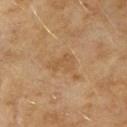Clinical impression:
The lesion was tiled from a total-body skin photograph and was not biopsied.
Acquisition and patient details:
From the right upper arm. The tile uses cross-polarized illumination. A 15 mm close-up tile from a total-body photography series done for melanoma screening. A female subject, roughly 60 years of age. Approximately 3.5 mm at its widest.